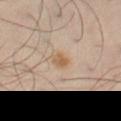workup: imaged on a skin check; not biopsied
anatomic site: the lower back
illumination: cross-polarized illumination
subject: male, about 50 years old
size: ~2.5 mm (longest diameter)
image source: 15 mm crop, total-body photography
image-analysis metrics: an area of roughly 4 mm², a shape eccentricity near 0.7, and two-axis asymmetry of about 0.3; a mean CIELAB color near L≈58 a*≈15 b*≈32, a lesion–skin lightness drop of about 7, and a lesion-to-skin contrast of about 7 (normalized; higher = more distinct); a border-irregularity rating of about 2.5/10, internal color variation of about 4.5 on a 0–10 scale, and peripheral color asymmetry of about 1.5; an automated nevus-likeness rating near 70 out of 100 and a detector confidence of about 100 out of 100 that the crop contains a lesion